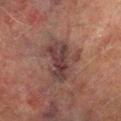biopsy status: catalogued during a skin exam; not biopsied | acquisition: ~15 mm crop, total-body skin-cancer survey | subject: male, in their mid-70s | location: the right lower leg | lighting: cross-polarized illumination | automated metrics: a mean CIELAB color near L≈32 a*≈16 b*≈17, about 8 CIELAB-L* units darker than the surrounding skin, and a normalized lesion–skin contrast near 8.5; a border-irregularity index near 5/10 and a color-variation rating of about 4.5/10 | diameter: ≈5.5 mm.